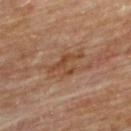No biopsy was performed on this lesion — it was imaged during a full skin examination and was not determined to be concerning. Imaged with cross-polarized lighting. Located on the upper back. The subject is a male aged around 85 or older. This image is a 15 mm lesion crop taken from a total-body photograph.